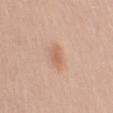The lesion was photographed on a routine skin check and not biopsied; there is no pathology result.
Imaged with white-light lighting.
Approximately 2.5 mm at its widest.
The lesion-visualizer software estimated a lesion color around L≈63 a*≈20 b*≈32 in CIELAB, roughly 8 lightness units darker than nearby skin, and a normalized border contrast of about 5.5.
A female subject aged 38 to 42.
Cropped from a total-body skin-imaging series; the visible field is about 15 mm.
On the left upper arm.